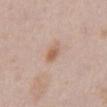{"biopsy_status": "not biopsied; imaged during a skin examination", "site": "abdomen", "image": {"source": "total-body photography crop", "field_of_view_mm": 15}, "lighting": "white-light", "lesion_size": {"long_diameter_mm_approx": 2.5}, "patient": {"sex": "male", "age_approx": 40}, "automated_metrics": {"eccentricity": 0.7, "shape_asymmetry": 0.15, "cielab_L": 60, "cielab_a": 19, "cielab_b": 30, "vs_skin_darker_L": 9.0, "nevus_likeness_0_100": 80, "lesion_detection_confidence_0_100": 100}}